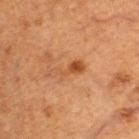<record>
  <biopsy_status>not biopsied; imaged during a skin examination</biopsy_status>
  <lesion_size>
    <long_diameter_mm_approx>4.0</long_diameter_mm_approx>
  </lesion_size>
  <automated_metrics>
    <area_mm2_approx>4.5</area_mm2_approx>
    <eccentricity>0.95</eccentricity>
    <cielab_L>40</cielab_L>
    <cielab_a>22</cielab_a>
    <cielab_b>32</cielab_b>
    <color_variation_0_10>1.5</color_variation_0_10>
    <peripheral_color_asymmetry>0.5</peripheral_color_asymmetry>
    <nevus_likeness_0_100>75</nevus_likeness_0_100>
    <lesion_detection_confidence_0_100>100</lesion_detection_confidence_0_100>
  </automated_metrics>
  <site>upper back</site>
  <patient>
    <sex>male</sex>
    <age_approx>50</age_approx>
  </patient>
  <image>
    <source>total-body photography crop</source>
    <field_of_view_mm>15</field_of_view_mm>
  </image>
</record>